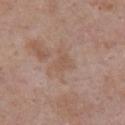This lesion was catalogued during total-body skin photography and was not selected for biopsy. A lesion tile, about 15 mm wide, cut from a 3D total-body photograph. This is a white-light tile. The lesion is on the chest. The subject is a male roughly 55 years of age. Automated tile analysis of the lesion measured radial color variation of about 0.5. And it measured a nevus-likeness score of about 0/100 and a detector confidence of about 100 out of 100 that the crop contains a lesion.